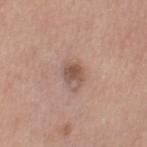  biopsy_status: not biopsied; imaged during a skin examination
  site: leg
  lesion_size:
    long_diameter_mm_approx: 2.5
  patient:
    sex: female
    age_approx: 45
  lighting: white-light
  automated_metrics:
    eccentricity: 0.6
    shape_asymmetry: 0.25
  image:
    source: total-body photography crop
    field_of_view_mm: 15